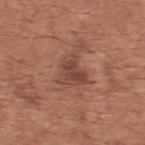Clinical impression: Captured during whole-body skin photography for melanoma surveillance; the lesion was not biopsied. Background: Longest diameter approximately 3.5 mm. A male patient, in their mid-60s. The lesion is on the upper back. Captured under white-light illumination. A roughly 15 mm field-of-view crop from a total-body skin photograph. The total-body-photography lesion software estimated roughly 9 lightness units darker than nearby skin and a lesion-to-skin contrast of about 7 (normalized; higher = more distinct).